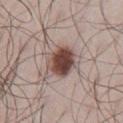Captured during whole-body skin photography for melanoma surveillance; the lesion was not biopsied.
Approximately 4 mm at its widest.
Cropped from a total-body skin-imaging series; the visible field is about 15 mm.
A male subject approximately 45 years of age.
The lesion is located on the abdomen.
Automated image analysis of the tile measured an area of roughly 11 mm², an eccentricity of roughly 0.4, and a symmetry-axis asymmetry near 0.15. The analysis additionally found border irregularity of about 1.5 on a 0–10 scale, a color-variation rating of about 7/10, and peripheral color asymmetry of about 2. It also reported a nevus-likeness score of about 100/100.